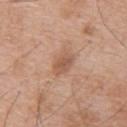Imaged during a routine full-body skin examination; the lesion was not biopsied and no histopathology is available. The subject is a male aged 63–67. This is a white-light tile. A roughly 15 mm field-of-view crop from a total-body skin photograph. The lesion is on the back. Automated image analysis of the tile measured a lesion–skin lightness drop of about 9 and a normalized border contrast of about 6.5. And it measured a border-irregularity rating of about 2.5/10, internal color variation of about 2.5 on a 0–10 scale, and a peripheral color-asymmetry measure near 1. The recorded lesion diameter is about 3 mm.